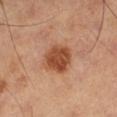Q: Was a biopsy performed?
A: imaged on a skin check; not biopsied
Q: What lighting was used for the tile?
A: cross-polarized illumination
Q: What is the lesion's diameter?
A: ~4 mm (longest diameter)
Q: What is the anatomic site?
A: the left lower leg
Q: What is the imaging modality?
A: ~15 mm crop, total-body skin-cancer survey
Q: What are the patient's age and sex?
A: male, approximately 60 years of age